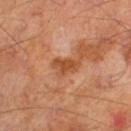follow-up: total-body-photography surveillance lesion; no biopsy
lighting: cross-polarized illumination
patient: male, aged 68 to 72
image source: ~15 mm tile from a whole-body skin photo
site: the right lower leg
lesion diameter: ~3 mm (longest diameter)